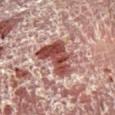Notes:
- workup — no biopsy performed (imaged during a skin exam)
- subject — male, aged 53–57
- imaging modality — total-body-photography crop, ~15 mm field of view
- site — the right lower leg
- lesion size — ≈5.5 mm
- lighting — cross-polarized